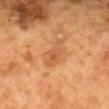{
  "biopsy_status": "not biopsied; imaged during a skin examination",
  "automated_metrics": {
    "area_mm2_approx": 6.0,
    "eccentricity": 0.9,
    "shape_asymmetry": 0.4,
    "nevus_likeness_0_100": 5,
    "lesion_detection_confidence_0_100": 100
  },
  "patient": {
    "sex": "female",
    "age_approx": 50
  },
  "lighting": "cross-polarized",
  "site": "mid back",
  "image": {
    "source": "total-body photography crop",
    "field_of_view_mm": 15
  }
}